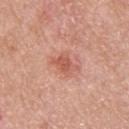- workup · no biopsy performed (imaged during a skin exam)
- patient · male, in their 80s
- automated lesion analysis · an area of roughly 5 mm², an eccentricity of roughly 0.7, and a shape-asymmetry score of about 0.35 (0 = symmetric); an average lesion color of about L≈57 a*≈28 b*≈31 (CIELAB), roughly 10 lightness units darker than nearby skin, and a normalized border contrast of about 6.5; a classifier nevus-likeness of about 65/100 and a detector confidence of about 100 out of 100 that the crop contains a lesion
- lesion diameter · ≈3 mm
- tile lighting · white-light
- location · the upper back
- acquisition · total-body-photography crop, ~15 mm field of view